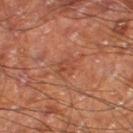<tbp_lesion>
  <lesion_size>
    <long_diameter_mm_approx>2.5</long_diameter_mm_approx>
  </lesion_size>
  <patient>
    <sex>male</sex>
    <age_approx>60</age_approx>
  </patient>
  <site>leg</site>
  <image>
    <source>total-body photography crop</source>
    <field_of_view_mm>15</field_of_view_mm>
  </image>
  <lighting>cross-polarized</lighting>
</tbp_lesion>